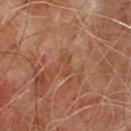Imaged during a routine full-body skin examination; the lesion was not biopsied and no histopathology is available.
A male patient, in their mid-60s.
The total-body-photography lesion software estimated a footprint of about 3 mm² and two-axis asymmetry of about 0.4. The software also gave a lesion–skin lightness drop of about 6 and a lesion-to-skin contrast of about 5.5 (normalized; higher = more distinct). The software also gave a color-variation rating of about 0/10 and a peripheral color-asymmetry measure near 0.
Longest diameter approximately 2.5 mm.
Imaged with cross-polarized lighting.
A region of skin cropped from a whole-body photographic capture, roughly 15 mm wide.